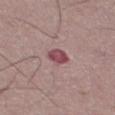Recorded during total-body skin imaging; not selected for excision or biopsy.
Captured under white-light illumination.
Measured at roughly 3 mm in maximum diameter.
A male subject in their mid-50s.
A roughly 15 mm field-of-view crop from a total-body skin photograph.